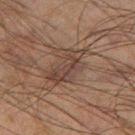Part of a total-body skin-imaging series; this lesion was reviewed on a skin check and was not flagged for biopsy. The tile uses cross-polarized illumination. Approximately 5 mm at its widest. The patient is a male about 65 years old. A 15 mm close-up extracted from a 3D total-body photography capture. On the left thigh.